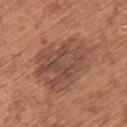{"biopsy_status": "not biopsied; imaged during a skin examination", "lesion_size": {"long_diameter_mm_approx": 8.0}, "patient": {"sex": "female", "age_approx": 65}, "lighting": "white-light", "automated_metrics": {"eccentricity": 0.7, "shape_asymmetry": 0.25, "vs_skin_darker_L": 9.0, "vs_skin_contrast_norm": 7.0, "lesion_detection_confidence_0_100": 100}, "site": "upper back", "image": {"source": "total-body photography crop", "field_of_view_mm": 15}}